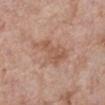<case>
  <biopsy_status>not biopsied; imaged during a skin examination</biopsy_status>
  <lesion_size>
    <long_diameter_mm_approx>6.0</long_diameter_mm_approx>
  </lesion_size>
  <patient>
    <sex>female</sex>
    <age_approx>70</age_approx>
  </patient>
  <image>
    <source>total-body photography crop</source>
    <field_of_view_mm>15</field_of_view_mm>
  </image>
  <lighting>white-light</lighting>
  <automated_metrics>
    <cielab_L>56</cielab_L>
    <cielab_a>20</cielab_a>
    <cielab_b>30</cielab_b>
    <vs_skin_darker_L>8.0</vs_skin_darker_L>
    <color_variation_0_10>3.0</color_variation_0_10>
  </automated_metrics>
  <site>left lower leg</site>
</case>